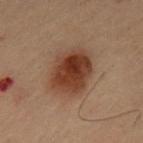follow-up: total-body-photography surveillance lesion; no biopsy
subject: male, in their mid-50s
automated lesion analysis: a footprint of about 19 mm², an outline eccentricity of about 0.65 (0 = round, 1 = elongated), and a symmetry-axis asymmetry near 0.15; a mean CIELAB color near L≈31 a*≈18 b*≈25, roughly 11 lightness units darker than nearby skin, and a normalized lesion–skin contrast near 10.5; a border-irregularity index near 2/10 and a within-lesion color-variation index near 5.5/10; a classifier nevus-likeness of about 100/100 and lesion-presence confidence of about 100/100
size: ≈5.5 mm
image: ~15 mm crop, total-body skin-cancer survey
tile lighting: cross-polarized
location: the mid back